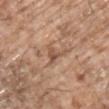| feature | finding |
|---|---|
| follow-up | catalogued during a skin exam; not biopsied |
| subject | male, aged 63–67 |
| lesion size | ≈3.5 mm |
| anatomic site | the chest |
| automated metrics | a mean CIELAB color near L≈53 a*≈19 b*≈31 and a normalized lesion–skin contrast near 6.5; a within-lesion color-variation index near 3/10; a classifier nevus-likeness of about 0/100 and a lesion-detection confidence of about 60/100 |
| illumination | white-light |
| imaging modality | ~15 mm tile from a whole-body skin photo |